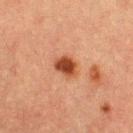Recorded during total-body skin imaging; not selected for excision or biopsy. A 15 mm close-up extracted from a 3D total-body photography capture. The tile uses cross-polarized illumination. Located on the arm. Approximately 3 mm at its widest. The lesion-visualizer software estimated an area of roughly 5.5 mm² and two-axis asymmetry of about 0.25. The analysis additionally found a nevus-likeness score of about 95/100 and lesion-presence confidence of about 100/100. A female patient, roughly 55 years of age.